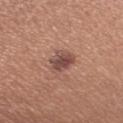Imaged during a routine full-body skin examination; the lesion was not biopsied and no histopathology is available.
Imaged with white-light lighting.
A 15 mm close-up extracted from a 3D total-body photography capture.
The patient is a female roughly 35 years of age.
On the left upper arm.
Measured at roughly 3 mm in maximum diameter.